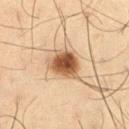Q: Was this lesion biopsied?
A: imaged on a skin check; not biopsied